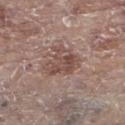Q: Was this lesion biopsied?
A: imaged on a skin check; not biopsied
Q: Illumination type?
A: white-light illumination
Q: How was this image acquired?
A: ~15 mm crop, total-body skin-cancer survey
Q: What is the anatomic site?
A: the left lower leg
Q: What are the patient's age and sex?
A: female, in their mid- to late 80s or older